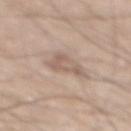Clinical impression:
Part of a total-body skin-imaging series; this lesion was reviewed on a skin check and was not flagged for biopsy.
Image and clinical context:
Captured under white-light illumination. An algorithmic analysis of the crop reported a shape eccentricity near 0.6. The analysis additionally found a normalized lesion–skin contrast near 5.5. A lesion tile, about 15 mm wide, cut from a 3D total-body photograph. A male subject, in their mid- to late 60s. The lesion is located on the mid back. Longest diameter approximately 4 mm.